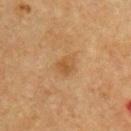Part of a total-body skin-imaging series; this lesion was reviewed on a skin check and was not flagged for biopsy.